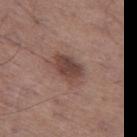Impression: No biopsy was performed on this lesion — it was imaged during a full skin examination and was not determined to be concerning. Image and clinical context: The tile uses white-light illumination. Measured at roughly 4 mm in maximum diameter. The lesion is located on the leg. The patient is a male about 70 years old. A 15 mm close-up extracted from a 3D total-body photography capture.